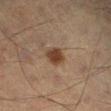No biopsy was performed on this lesion — it was imaged during a full skin examination and was not determined to be concerning. Located on the leg. The total-body-photography lesion software estimated a border-irregularity index near 1.5/10, a within-lesion color-variation index near 3.5/10, and radial color variation of about 1. The analysis additionally found a nevus-likeness score of about 95/100 and a detector confidence of about 100 out of 100 that the crop contains a lesion. Cropped from a whole-body photographic skin survey; the tile spans about 15 mm. A male subject aged 58–62. The tile uses cross-polarized illumination.